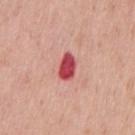follow-up — catalogued during a skin exam; not biopsied
subject — male, roughly 60 years of age
image-analysis metrics — a lesion color around L≈50 a*≈42 b*≈25 in CIELAB and roughly 19 lightness units darker than nearby skin; a border-irregularity index near 2.5/10, a color-variation rating of about 2.5/10, and radial color variation of about 1; a classifier nevus-likeness of about 0/100
body site — the chest
imaging modality — ~15 mm tile from a whole-body skin photo
size — about 3 mm
illumination — white-light illumination